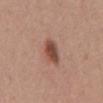Q: Is there a histopathology result?
A: catalogued during a skin exam; not biopsied
Q: How was this image acquired?
A: 15 mm crop, total-body photography
Q: Lesion size?
A: ~3.5 mm (longest diameter)
Q: Who is the patient?
A: male, aged approximately 30
Q: How was the tile lit?
A: white-light
Q: Lesion location?
A: the mid back
Q: Automated lesion metrics?
A: an area of roughly 6.5 mm², an outline eccentricity of about 0.8 (0 = round, 1 = elongated), and two-axis asymmetry of about 0.2; a border-irregularity index near 2/10 and a within-lesion color-variation index near 4.5/10